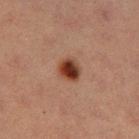No biopsy was performed on this lesion — it was imaged during a full skin examination and was not determined to be concerning.
Located on the left thigh.
Cropped from a total-body skin-imaging series; the visible field is about 15 mm.
The recorded lesion diameter is about 2.5 mm.
The patient is a female about 40 years old.
The total-body-photography lesion software estimated a lesion area of about 6 mm², an outline eccentricity of about 0.55 (0 = round, 1 = elongated), and two-axis asymmetry of about 0.2. It also reported an average lesion color of about L≈32 a*≈21 b*≈26 (CIELAB), about 13 CIELAB-L* units darker than the surrounding skin, and a normalized border contrast of about 12. And it measured a border-irregularity rating of about 1.5/10 and a peripheral color-asymmetry measure near 2.
The tile uses cross-polarized illumination.